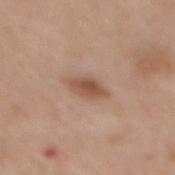biopsy_status: not biopsied; imaged during a skin examination
patient:
  sex: male
  age_approx: 65
image:
  source: total-body photography crop
  field_of_view_mm: 15
site: mid back